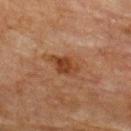<record>
<biopsy_status>not biopsied; imaged during a skin examination</biopsy_status>
<image>
  <source>total-body photography crop</source>
  <field_of_view_mm>15</field_of_view_mm>
</image>
<lighting>cross-polarized</lighting>
<patient>
  <sex>female</sex>
  <age_approx>80</age_approx>
</patient>
<lesion_size>
  <long_diameter_mm_approx>4.0</long_diameter_mm_approx>
</lesion_size>
<automated_metrics>
  <border_irregularity_0_10>3.0</border_irregularity_0_10>
  <peripheral_color_asymmetry>1.5</peripheral_color_asymmetry>
  <nevus_likeness_0_100>85</nevus_likeness_0_100>
</automated_metrics>
<site>back</site>
</record>